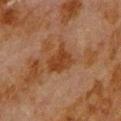A male subject, aged around 80. From the upper back. A region of skin cropped from a whole-body photographic capture, roughly 15 mm wide. An algorithmic analysis of the crop reported a footprint of about 7.5 mm², a shape eccentricity near 0.3, and two-axis asymmetry of about 0.35. It also reported a mean CIELAB color near L≈31 a*≈19 b*≈30 and roughly 7 lightness units darker than nearby skin. And it measured border irregularity of about 3.5 on a 0–10 scale, a color-variation rating of about 2.5/10, and a peripheral color-asymmetry measure near 1. The analysis additionally found a nevus-likeness score of about 0/100 and a lesion-detection confidence of about 100/100. Measured at roughly 3.5 mm in maximum diameter.